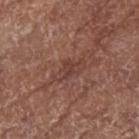workup = total-body-photography surveillance lesion; no biopsy | lesion size = about 4.5 mm | acquisition = 15 mm crop, total-body photography | lighting = white-light illumination | subject = female, about 75 years old | body site = the right forearm.